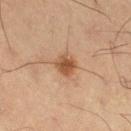Clinical impression: No biopsy was performed on this lesion — it was imaged during a full skin examination and was not determined to be concerning. Context: This is a cross-polarized tile. A male patient aged approximately 65. The lesion-visualizer software estimated a lesion color around L≈42 a*≈18 b*≈29 in CIELAB, about 10 CIELAB-L* units darker than the surrounding skin, and a lesion-to-skin contrast of about 8.5 (normalized; higher = more distinct). It also reported border irregularity of about 1.5 on a 0–10 scale and peripheral color asymmetry of about 1. Located on the right thigh. The lesion's longest dimension is about 2.5 mm. A roughly 15 mm field-of-view crop from a total-body skin photograph.